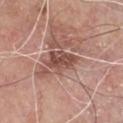A region of skin cropped from a whole-body photographic capture, roughly 15 mm wide. The total-body-photography lesion software estimated an area of roughly 8 mm², an eccentricity of roughly 0.8, and a symmetry-axis asymmetry near 0.3. It also reported an average lesion color of about L≈49 a*≈22 b*≈25 (CIELAB), about 12 CIELAB-L* units darker than the surrounding skin, and a normalized border contrast of about 8.5. A male subject, aged around 80. On the chest. Longest diameter approximately 4.5 mm. Imaged with white-light lighting.